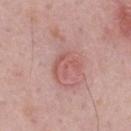This lesion was catalogued during total-body skin photography and was not selected for biopsy. The total-body-photography lesion software estimated a lesion color around L≈57 a*≈26 b*≈24 in CIELAB and a normalized lesion–skin contrast near 5.5. A close-up tile cropped from a whole-body skin photograph, about 15 mm across. The subject is a male aged around 50. On the mid back. The tile uses white-light illumination. The recorded lesion diameter is about 3.5 mm.